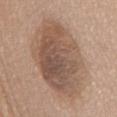follow-up: total-body-photography surveillance lesion; no biopsy | subject: female, aged around 60 | tile lighting: white-light illumination | image-analysis metrics: an area of roughly 50 mm² and an outline eccentricity of about 0.8 (0 = round, 1 = elongated); a border-irregularity index near 1.5/10, internal color variation of about 6.5 on a 0–10 scale, and radial color variation of about 2 | size: ~11 mm (longest diameter) | image: 15 mm crop, total-body photography | anatomic site: the mid back.